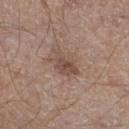| feature | finding |
|---|---|
| acquisition | ~15 mm tile from a whole-body skin photo |
| anatomic site | the right lower leg |
| image-analysis metrics | an area of roughly 7 mm², a shape eccentricity near 0.75, and a shape-asymmetry score of about 0.3 (0 = symmetric); a lesion color around L≈48 a*≈17 b*≈24 in CIELAB and a lesion-to-skin contrast of about 7 (normalized; higher = more distinct); a color-variation rating of about 3.5/10 and radial color variation of about 1.5; a nevus-likeness score of about 40/100 |
| lesion diameter | about 4 mm |
| patient | male, roughly 60 years of age |
| lighting | white-light |